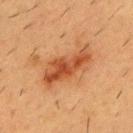Imaged during a routine full-body skin examination; the lesion was not biopsied and no histopathology is available. Located on the chest. Automated tile analysis of the lesion measured a footprint of about 14 mm², an eccentricity of roughly 0.9, and two-axis asymmetry of about 0.3. A male subject approximately 55 years of age. About 6.5 mm across. A 15 mm close-up tile from a total-body photography series done for melanoma screening. Captured under cross-polarized illumination.